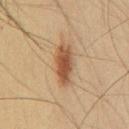| field | value |
|---|---|
| workup | catalogued during a skin exam; not biopsied |
| illumination | cross-polarized |
| patient | male, aged 33 to 37 |
| body site | the back |
| lesion diameter | ≈4.5 mm |
| imaging modality | total-body-photography crop, ~15 mm field of view |